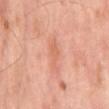Located on the mid back.
Cropped from a whole-body photographic skin survey; the tile spans about 15 mm.
A male patient, aged 63–67.
Imaged with cross-polarized lighting.